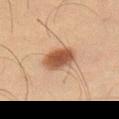Case summary:
• notes · no biopsy performed (imaged during a skin exam)
• location · the right thigh
• subject · male, in their mid- to late 40s
• tile lighting · cross-polarized
• acquisition · total-body-photography crop, ~15 mm field of view